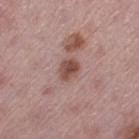Imaged with white-light lighting.
Measured at roughly 2.5 mm in maximum diameter.
A close-up tile cropped from a whole-body skin photograph, about 15 mm across.
Automated tile analysis of the lesion measured a lesion area of about 4.5 mm², an eccentricity of roughly 0.65, and a symmetry-axis asymmetry near 0.15. The analysis additionally found a lesion color around L≈48 a*≈21 b*≈23 in CIELAB and a normalized border contrast of about 9. The software also gave a border-irregularity index near 1.5/10, a color-variation rating of about 2.5/10, and a peripheral color-asymmetry measure near 1. The analysis additionally found an automated nevus-likeness rating near 65 out of 100.
On the left thigh.
A female patient aged 43–47.